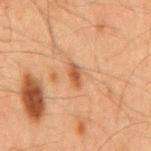Recorded during total-body skin imaging; not selected for excision or biopsy. The recorded lesion diameter is about 2.5 mm. On the mid back. A male patient in their mid-60s. A region of skin cropped from a whole-body photographic capture, roughly 15 mm wide.